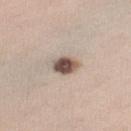- follow-up: imaged on a skin check; not biopsied